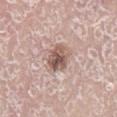{
  "biopsy_status": "not biopsied; imaged during a skin examination",
  "patient": {
    "sex": "male",
    "age_approx": 80
  },
  "site": "right lower leg",
  "image": {
    "source": "total-body photography crop",
    "field_of_view_mm": 15
  },
  "automated_metrics": {
    "eccentricity": 0.45,
    "shape_asymmetry": 0.15,
    "cielab_L": 55,
    "cielab_a": 18,
    "cielab_b": 23,
    "vs_skin_darker_L": 14.0,
    "vs_skin_contrast_norm": 9.0,
    "nevus_likeness_0_100": 20,
    "lesion_detection_confidence_0_100": 100
  }
}